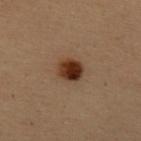Clinical impression:
Imaged during a routine full-body skin examination; the lesion was not biopsied and no histopathology is available.
Acquisition and patient details:
The lesion is on the upper back. A female patient, aged around 50. This image is a 15 mm lesion crop taken from a total-body photograph.